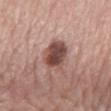biopsy status: total-body-photography surveillance lesion; no biopsy